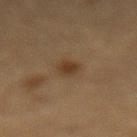biopsy_status: not biopsied; imaged during a skin examination
automated_metrics:
  area_mm2_approx: 5.0
  cielab_L: 32
  cielab_a: 13
  cielab_b: 26
  vs_skin_darker_L: 7.0
  vs_skin_contrast_norm: 7.0
  border_irregularity_0_10: 2.0
  color_variation_0_10: 2.5
lighting: cross-polarized
lesion_size:
  long_diameter_mm_approx: 3.0
site: lower back
image:
  source: total-body photography crop
  field_of_view_mm: 15
patient:
  sex: male
  age_approx: 85+